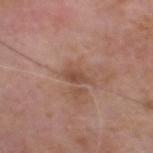<case>
  <lesion_size>
    <long_diameter_mm_approx>3.0</long_diameter_mm_approx>
  </lesion_size>
  <lighting>white-light</lighting>
  <automated_metrics>
    <cielab_L>48</cielab_L>
    <cielab_a>20</cielab_a>
    <cielab_b>28</cielab_b>
    <vs_skin_darker_L>8.0</vs_skin_darker_L>
    <vs_skin_contrast_norm>6.5</vs_skin_contrast_norm>
  </automated_metrics>
  <patient>
    <sex>male</sex>
    <age_approx>65</age_approx>
  </patient>
  <site>head or neck</site>
  <image>
    <source>total-body photography crop</source>
    <field_of_view_mm>15</field_of_view_mm>
  </image>
</case>